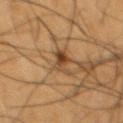<tbp_lesion>
  <biopsy_status>not biopsied; imaged during a skin examination</biopsy_status>
  <site>chest</site>
  <lesion_size>
    <long_diameter_mm_approx>3.5</long_diameter_mm_approx>
  </lesion_size>
  <image>
    <source>total-body photography crop</source>
    <field_of_view_mm>15</field_of_view_mm>
  </image>
  <lighting>cross-polarized</lighting>
  <patient>
    <sex>male</sex>
    <age_approx>55</age_approx>
  </patient>
</tbp_lesion>